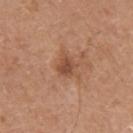workup = imaged on a skin check; not biopsied.